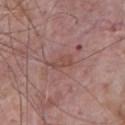Q: Is there a histopathology result?
A: catalogued during a skin exam; not biopsied
Q: What did automated image analysis measure?
A: two-axis asymmetry of about 0.3; an average lesion color of about L≈48 a*≈22 b*≈23 (CIELAB), a lesion–skin lightness drop of about 6, and a lesion-to-skin contrast of about 5.5 (normalized; higher = more distinct); a border-irregularity rating of about 3.5/10, a color-variation rating of about 0/10, and radial color variation of about 0
Q: How large is the lesion?
A: about 2.5 mm
Q: What is the imaging modality?
A: 15 mm crop, total-body photography
Q: Who is the patient?
A: male, approximately 75 years of age
Q: Where on the body is the lesion?
A: the chest